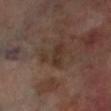This lesion was catalogued during total-body skin photography and was not selected for biopsy. The subject is a male in their 70s. About 4 mm across. Located on the left lower leg. A 15 mm close-up tile from a total-body photography series done for melanoma screening. The tile uses cross-polarized illumination.